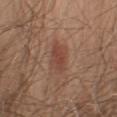The lesion was tiled from a total-body skin photograph and was not biopsied. The lesion is located on the right upper arm. Longest diameter approximately 4.5 mm. A 15 mm close-up tile from a total-body photography series done for melanoma screening. This is a white-light tile. A male subject about 55 years old. The total-body-photography lesion software estimated a shape eccentricity near 0.85 and a shape-asymmetry score of about 0.25 (0 = symmetric). And it measured a classifier nevus-likeness of about 85/100 and a detector confidence of about 100 out of 100 that the crop contains a lesion.